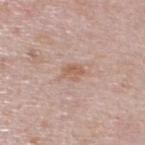The lesion was tiled from a total-body skin photograph and was not biopsied. Located on the upper back. Automated image analysis of the tile measured an automated nevus-likeness rating near 10 out of 100. The tile uses white-light illumination. A 15 mm close-up tile from a total-body photography series done for melanoma screening. A male subject, about 40 years old.